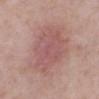The lesion was photographed on a routine skin check and not biopsied; there is no pathology result.
Longest diameter approximately 7.5 mm.
Cropped from a whole-body photographic skin survey; the tile spans about 15 mm.
A female subject, roughly 50 years of age.
Imaged with white-light lighting.
On the leg.